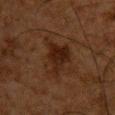The tile uses cross-polarized illumination.
The lesion's longest dimension is about 4.5 mm.
A male subject in their mid- to late 60s.
On the chest.
The lesion-visualizer software estimated a lesion color around L≈18 a*≈16 b*≈22 in CIELAB, about 6 CIELAB-L* units darker than the surrounding skin, and a lesion-to-skin contrast of about 8.5 (normalized; higher = more distinct).
This image is a 15 mm lesion crop taken from a total-body photograph.